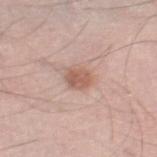Q: Is there a histopathology result?
A: catalogued during a skin exam; not biopsied
Q: What lighting was used for the tile?
A: white-light
Q: Lesion location?
A: the leg
Q: What are the patient's age and sex?
A: male, about 55 years old
Q: What did automated image analysis measure?
A: an average lesion color of about L≈59 a*≈21 b*≈27 (CIELAB), a lesion–skin lightness drop of about 10, and a normalized lesion–skin contrast near 7; a border-irregularity rating of about 2/10 and a within-lesion color-variation index near 2.5/10
Q: What kind of image is this?
A: total-body-photography crop, ~15 mm field of view
Q: How large is the lesion?
A: about 2.5 mm